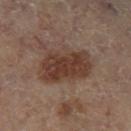Findings:
- notes · catalogued during a skin exam; not biopsied
- illumination · cross-polarized illumination
- patient · female, aged approximately 60
- imaging modality · ~15 mm tile from a whole-body skin photo
- lesion diameter · ~6.5 mm (longest diameter)
- location · the left lower leg